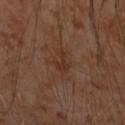This lesion was catalogued during total-body skin photography and was not selected for biopsy. A lesion tile, about 15 mm wide, cut from a 3D total-body photograph. A male subject, aged 53 to 57. On the left forearm.